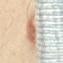Context: The recorded lesion diameter is about 6.5 mm. On the lower back. A close-up tile cropped from a whole-body skin photograph, about 15 mm across. A female patient, aged approximately 40.